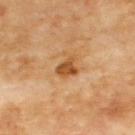workup: no biopsy performed (imaged during a skin exam) | patient: male, aged 68–72 | image-analysis metrics: an area of roughly 5 mm², an outline eccentricity of about 0.5 (0 = round, 1 = elongated), and a shape-asymmetry score of about 0.35 (0 = symmetric); a lesion–skin lightness drop of about 12 and a lesion-to-skin contrast of about 9 (normalized; higher = more distinct); a border-irregularity rating of about 3.5/10, internal color variation of about 2.5 on a 0–10 scale, and a peripheral color-asymmetry measure near 1 | site: the upper back | imaging modality: total-body-photography crop, ~15 mm field of view.